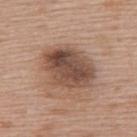Assessment: Part of a total-body skin-imaging series; this lesion was reviewed on a skin check and was not flagged for biopsy. Clinical summary: Imaged with white-light lighting. The subject is a female aged 58–62. On the back. A 15 mm crop from a total-body photograph taken for skin-cancer surveillance.